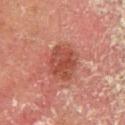No biopsy was performed on this lesion — it was imaged during a full skin examination and was not determined to be concerning.
About 4 mm across.
A 15 mm close-up extracted from a 3D total-body photography capture.
A male subject, in their 80s.
Imaged with cross-polarized lighting.
Automated image analysis of the tile measured an area of roughly 11 mm², a shape eccentricity near 0.55, and two-axis asymmetry of about 0.2. The analysis additionally found a mean CIELAB color near L≈36 a*≈24 b*≈25, a lesion–skin lightness drop of about 8, and a normalized lesion–skin contrast near 7.5. The software also gave border irregularity of about 2 on a 0–10 scale, internal color variation of about 3 on a 0–10 scale, and radial color variation of about 1. It also reported a nevus-likeness score of about 10/100 and a lesion-detection confidence of about 100/100.
The lesion is on the leg.